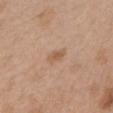Recorded during total-body skin imaging; not selected for excision or biopsy.
The tile uses white-light illumination.
The patient is a male approximately 55 years of age.
From the chest.
About 2.5 mm across.
The lesion-visualizer software estimated a footprint of about 3 mm², an eccentricity of roughly 0.85, and a symmetry-axis asymmetry near 0.35. The software also gave a mean CIELAB color near L≈57 a*≈19 b*≈32 and roughly 8 lightness units darker than nearby skin.
Cropped from a total-body skin-imaging series; the visible field is about 15 mm.